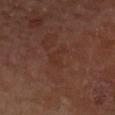<tbp_lesion>
<biopsy_status>not biopsied; imaged during a skin examination</biopsy_status>
<image>
  <source>total-body photography crop</source>
  <field_of_view_mm>15</field_of_view_mm>
</image>
<site>left forearm</site>
<patient>
  <sex>female</sex>
  <age_approx>70</age_approx>
</patient>
<lighting>cross-polarized</lighting>
</tbp_lesion>